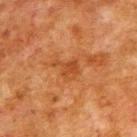Case summary:
• imaging modality: 15 mm crop, total-body photography
• patient: male, about 80 years old
• site: the upper back